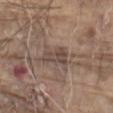{
  "biopsy_status": "not biopsied; imaged during a skin examination",
  "patient": {
    "sex": "male",
    "age_approx": 80
  },
  "lighting": "white-light",
  "lesion_size": {
    "long_diameter_mm_approx": 3.5
  },
  "image": {
    "source": "total-body photography crop",
    "field_of_view_mm": 15
  },
  "site": "abdomen"
}